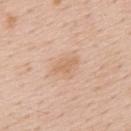site: the upper back | automated lesion analysis: a footprint of about 3.5 mm² and a shape eccentricity near 0.9; a mean CIELAB color near L≈65 a*≈20 b*≈34 and a normalized border contrast of about 5.5; an automated nevus-likeness rating near 5 out of 100 and a lesion-detection confidence of about 100/100 | subject: male, aged around 60 | lighting: white-light illumination | lesion diameter: ~3 mm (longest diameter) | image: ~15 mm crop, total-body skin-cancer survey.